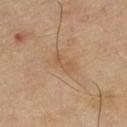Impression: No biopsy was performed on this lesion — it was imaged during a full skin examination and was not determined to be concerning. Clinical summary: A female patient, aged 68 to 72. The lesion-visualizer software estimated a footprint of about 3 mm², a shape eccentricity near 0.9, and a symmetry-axis asymmetry near 0.35. It also reported a lesion color around L≈56 a*≈19 b*≈35 in CIELAB, roughly 6 lightness units darker than nearby skin, and a lesion-to-skin contrast of about 5 (normalized; higher = more distinct). And it measured a border-irregularity rating of about 4.5/10 and a peripheral color-asymmetry measure near 0.5. The software also gave an automated nevus-likeness rating near 0 out of 100 and a lesion-detection confidence of about 100/100. A 15 mm close-up extracted from a 3D total-body photography capture. About 3 mm across. Located on the right lower leg.